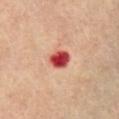Assessment: The lesion was photographed on a routine skin check and not biopsied; there is no pathology result. Context: Longest diameter approximately 3 mm. A roughly 15 mm field-of-view crop from a total-body skin photograph. The patient is a male about 65 years old. Located on the chest. This is a cross-polarized tile.